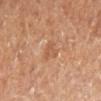Q: Is there a histopathology result?
A: catalogued during a skin exam; not biopsied